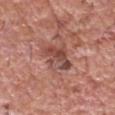Recorded during total-body skin imaging; not selected for excision or biopsy. Automated image analysis of the tile measured a mean CIELAB color near L≈47 a*≈24 b*≈26, about 10 CIELAB-L* units darker than the surrounding skin, and a normalized lesion–skin contrast near 7.5. The analysis additionally found a border-irregularity index near 4/10, a within-lesion color-variation index near 7.5/10, and peripheral color asymmetry of about 2. Cropped from a whole-body photographic skin survey; the tile spans about 15 mm. On the head or neck. The tile uses white-light illumination. A male subject, aged 58 to 62. Longest diameter approximately 5.5 mm.